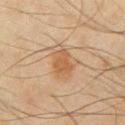Q: Is there a histopathology result?
A: total-body-photography surveillance lesion; no biopsy
Q: What did automated image analysis measure?
A: an eccentricity of roughly 0.7 and two-axis asymmetry of about 0.25
Q: What is the anatomic site?
A: the chest
Q: What is the lesion's diameter?
A: ≈3 mm
Q: What are the patient's age and sex?
A: male, about 45 years old
Q: How was this image acquired?
A: ~15 mm tile from a whole-body skin photo